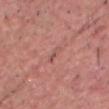biopsy_status: not biopsied; imaged during a skin examination
patient:
  sex: male
  age_approx: 60
site: head or neck
lighting: white-light
image:
  source: total-body photography crop
  field_of_view_mm: 15